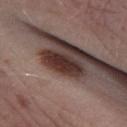Q: Was this lesion biopsied?
A: no biopsy performed (imaged during a skin exam)
Q: Automated lesion metrics?
A: border irregularity of about 2.5 on a 0–10 scale and internal color variation of about 3 on a 0–10 scale
Q: Where on the body is the lesion?
A: the leg
Q: What is the imaging modality?
A: ~15 mm crop, total-body skin-cancer survey
Q: Illumination type?
A: white-light illumination
Q: Patient demographics?
A: male, in their mid- to late 40s